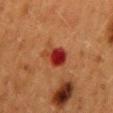Clinical impression:
This lesion was catalogued during total-body skin photography and was not selected for biopsy.
Background:
From the back. The subject is a female about 50 years old. The recorded lesion diameter is about 3 mm. The total-body-photography lesion software estimated a lesion color around L≈30 a*≈32 b*≈29 in CIELAB, roughly 13 lightness units darker than nearby skin, and a normalized border contrast of about 11.5. It also reported border irregularity of about 2.5 on a 0–10 scale, internal color variation of about 4.5 on a 0–10 scale, and peripheral color asymmetry of about 1.5. Cropped from a total-body skin-imaging series; the visible field is about 15 mm.